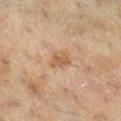workup = catalogued during a skin exam; not biopsied
image = 15 mm crop, total-body photography
location = the right lower leg
patient = female, about 60 years old
image-analysis metrics = a lesion area of about 4.5 mm², a shape eccentricity near 0.7, and a symmetry-axis asymmetry near 0.35; a mean CIELAB color near L≈52 a*≈18 b*≈31 and a lesion–skin lightness drop of about 9; a nevus-likeness score of about 15/100 and a lesion-detection confidence of about 100/100
illumination = cross-polarized